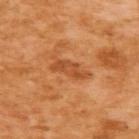Recorded during total-body skin imaging; not selected for excision or biopsy.
Located on the upper back.
Imaged with cross-polarized lighting.
Cropped from a total-body skin-imaging series; the visible field is about 15 mm.
The subject is a female aged around 55.
Longest diameter approximately 4.5 mm.
An algorithmic analysis of the crop reported a footprint of about 6.5 mm², a shape eccentricity near 0.9, and a shape-asymmetry score of about 0.3 (0 = symmetric). It also reported a mean CIELAB color near L≈52 a*≈29 b*≈43, about 9 CIELAB-L* units darker than the surrounding skin, and a lesion-to-skin contrast of about 6.5 (normalized; higher = more distinct). And it measured a border-irregularity index near 4/10, a within-lesion color-variation index near 4/10, and a peripheral color-asymmetry measure near 1.5. And it measured lesion-presence confidence of about 100/100.